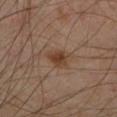Captured under cross-polarized illumination. A subject roughly 55 years of age. Measured at roughly 2.5 mm in maximum diameter. Cropped from a total-body skin-imaging series; the visible field is about 15 mm. Located on the left lower leg.